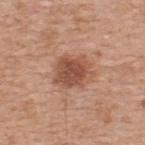biopsy_status: not biopsied; imaged during a skin examination
patient:
  sex: male
  age_approx: 55
lighting: white-light
automated_metrics:
  eccentricity: 0.5
  shape_asymmetry: 0.2
  cielab_L: 51
  cielab_a: 23
  cielab_b: 30
  vs_skin_darker_L: 12.0
  vs_skin_contrast_norm: 8.5
  border_irregularity_0_10: 2.5
  peripheral_color_asymmetry: 1.0
lesion_size:
  long_diameter_mm_approx: 4.0
site: upper back
image:
  source: total-body photography crop
  field_of_view_mm: 15